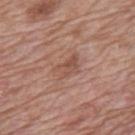  biopsy_status: not biopsied; imaged during a skin examination